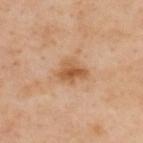follow-up = catalogued during a skin exam; not biopsied | tile lighting = cross-polarized | anatomic site = the upper back | image = 15 mm crop, total-body photography | lesion diameter = ~3.5 mm (longest diameter) | patient = male, aged around 55.